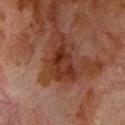Clinical impression:
No biopsy was performed on this lesion — it was imaged during a full skin examination and was not determined to be concerning.
Clinical summary:
Located on the chest. A male subject aged around 80. A 15 mm close-up tile from a total-body photography series done for melanoma screening. Automated tile analysis of the lesion measured a border-irregularity rating of about 5.5/10, a color-variation rating of about 4.5/10, and radial color variation of about 1.5. The analysis additionally found an automated nevus-likeness rating near 0 out of 100 and lesion-presence confidence of about 100/100. The recorded lesion diameter is about 5.5 mm. The tile uses cross-polarized illumination.